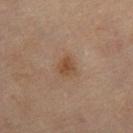- workup · no biopsy performed (imaged during a skin exam)
- subject · female, aged approximately 80
- tile lighting · cross-polarized illumination
- image source · total-body-photography crop, ~15 mm field of view
- site · the right thigh
- diameter · about 2.5 mm
- TBP lesion metrics · a footprint of about 5 mm², an eccentricity of roughly 0.5, and a symmetry-axis asymmetry near 0.25; a border-irregularity rating of about 2/10, a within-lesion color-variation index near 2.5/10, and a peripheral color-asymmetry measure near 1; a classifier nevus-likeness of about 50/100 and a lesion-detection confidence of about 100/100